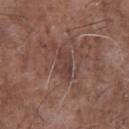{"image": {"source": "total-body photography crop", "field_of_view_mm": 15}, "site": "chest", "patient": {"sex": "male", "age_approx": 70}}